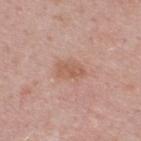Notes:
• follow-up — no biopsy performed (imaged during a skin exam)
• illumination — white-light illumination
• acquisition — ~15 mm crop, total-body skin-cancer survey
• automated metrics — an area of roughly 5.5 mm² and an outline eccentricity of about 0.8 (0 = round, 1 = elongated); a lesion–skin lightness drop of about 8 and a normalized border contrast of about 6
• subject — male, aged approximately 50
• anatomic site — the back